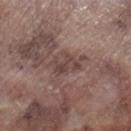- workup: no biopsy performed (imaged during a skin exam)
- lesion diameter: ~3 mm (longest diameter)
- imaging modality: ~15 mm crop, total-body skin-cancer survey
- site: the right lower leg
- subject: male, aged 73 to 77